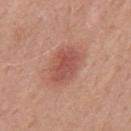Clinical impression: The lesion was photographed on a routine skin check and not biopsied; there is no pathology result. Acquisition and patient details: Located on the mid back. This is a white-light tile. A male subject, approximately 50 years of age. A 15 mm close-up tile from a total-body photography series done for melanoma screening. Approximately 5 mm at its widest.